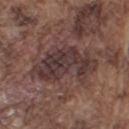Impression: Recorded during total-body skin imaging; not selected for excision or biopsy. Acquisition and patient details: Captured under white-light illumination. The lesion's longest dimension is about 7.5 mm. Located on the arm. A male patient in their mid-70s. Automated image analysis of the tile measured roughly 10 lightness units darker than nearby skin and a normalized border contrast of about 9.5. It also reported an automated nevus-likeness rating near 0 out of 100 and a detector confidence of about 70 out of 100 that the crop contains a lesion. Cropped from a whole-body photographic skin survey; the tile spans about 15 mm.